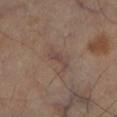notes: imaged on a skin check; not biopsied | subject: female, in their 60s | site: the right leg | illumination: cross-polarized illumination | TBP lesion metrics: a mean CIELAB color near L≈44 a*≈16 b*≈21 and a normalized lesion–skin contrast near 5.5; a nevus-likeness score of about 0/100 and a lesion-detection confidence of about 100/100 | imaging modality: 15 mm crop, total-body photography | size: ~3 mm (longest diameter).